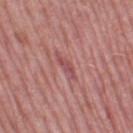Imaged during a routine full-body skin examination; the lesion was not biopsied and no histopathology is available. This image is a 15 mm lesion crop taken from a total-body photograph. A female subject aged 58–62. Located on the left thigh.